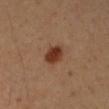This lesion was catalogued during total-body skin photography and was not selected for biopsy. The recorded lesion diameter is about 3 mm. On the right forearm. A female patient, about 35 years old. This image is a 15 mm lesion crop taken from a total-body photograph. The tile uses cross-polarized illumination. Automated tile analysis of the lesion measured a lesion color around L≈35 a*≈22 b*≈29 in CIELAB, roughly 12 lightness units darker than nearby skin, and a normalized border contrast of about 11. It also reported a border-irregularity index near 1.5/10, internal color variation of about 3 on a 0–10 scale, and a peripheral color-asymmetry measure near 1. The analysis additionally found an automated nevus-likeness rating near 100 out of 100.